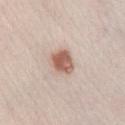The lesion was tiled from a total-body skin photograph and was not biopsied. The lesion is located on the right lower leg. A 15 mm close-up tile from a total-body photography series done for melanoma screening. Captured under white-light illumination. A male subject aged 23 to 27.